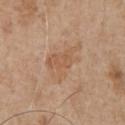– workup — no biopsy performed (imaged during a skin exam)
– tile lighting — white-light
– location — the chest
– image-analysis metrics — a footprint of about 11 mm², an outline eccentricity of about 0.8 (0 = round, 1 = elongated), and two-axis asymmetry of about 0.45; a mean CIELAB color near L≈57 a*≈19 b*≈33, about 6 CIELAB-L* units darker than the surrounding skin, and a normalized border contrast of about 5; border irregularity of about 5.5 on a 0–10 scale and peripheral color asymmetry of about 1
– subject — male, aged 63–67
– acquisition — ~15 mm tile from a whole-body skin photo
– diameter — about 5.5 mm